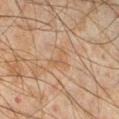Findings:
– follow-up · catalogued during a skin exam; not biopsied
– image · ~15 mm tile from a whole-body skin photo
– anatomic site · the right lower leg
– lesion diameter · about 2.5 mm
– subject · male, in their mid- to late 40s
– lighting · cross-polarized